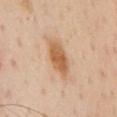Impression: This lesion was catalogued during total-body skin photography and was not selected for biopsy. Image and clinical context: A 15 mm close-up tile from a total-body photography series done for melanoma screening. A male subject, approximately 55 years of age. On the chest. Approximately 5.5 mm at its widest. The tile uses cross-polarized illumination.